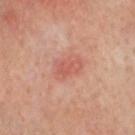Assessment: Part of a total-body skin-imaging series; this lesion was reviewed on a skin check and was not flagged for biopsy. Clinical summary: The lesion is on the head or neck. Imaged with cross-polarized lighting. The subject is a male aged 63–67. A 15 mm crop from a total-body photograph taken for skin-cancer surveillance. Approximately 3.5 mm at its widest.